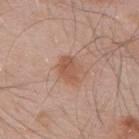Notes:
- follow-up — no biopsy performed (imaged during a skin exam)
- automated lesion analysis — a footprint of about 6 mm² and a shape-asymmetry score of about 0.2 (0 = symmetric); a mean CIELAB color near L≈54 a*≈22 b*≈30 and a normalized lesion–skin contrast near 7; border irregularity of about 2 on a 0–10 scale, a color-variation rating of about 2.5/10, and a peripheral color-asymmetry measure near 1
- image source — total-body-photography crop, ~15 mm field of view
- subject — male, aged approximately 55
- site — the mid back
- illumination — white-light illumination